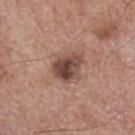Captured during whole-body skin photography for melanoma surveillance; the lesion was not biopsied. On the back. A region of skin cropped from a whole-body photographic capture, roughly 15 mm wide. Approximately 4 mm at its widest. A male subject in their mid-60s. Imaged with white-light lighting. The lesion-visualizer software estimated about 14 CIELAB-L* units darker than the surrounding skin.